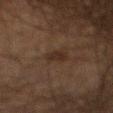Part of a total-body skin-imaging series; this lesion was reviewed on a skin check and was not flagged for biopsy.
A male patient, about 60 years old.
On the mid back.
A lesion tile, about 15 mm wide, cut from a 3D total-body photograph.
The tile uses cross-polarized illumination.